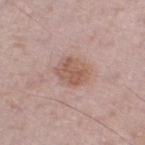<tbp_lesion>
  <biopsy_status>not biopsied; imaged during a skin examination</biopsy_status>
  <lighting>white-light</lighting>
  <image>
    <source>total-body photography crop</source>
    <field_of_view_mm>15</field_of_view_mm>
  </image>
  <automated_metrics>
    <nevus_likeness_0_100>15</nevus_likeness_0_100>
  </automated_metrics>
  <lesion_size>
    <long_diameter_mm_approx>4.0</long_diameter_mm_approx>
  </lesion_size>
  <patient>
    <sex>male</sex>
    <age_approx>75</age_approx>
  </patient>
  <site>left thigh</site>
</tbp_lesion>